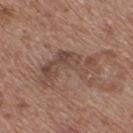Case summary:
– notes · no biopsy performed (imaged during a skin exam)
– body site · the right thigh
– subject · female, in their mid- to late 70s
– image · total-body-photography crop, ~15 mm field of view